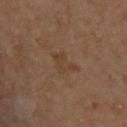  biopsy_status: not biopsied; imaged during a skin examination
  image:
    source: total-body photography crop
    field_of_view_mm: 15
  lighting: cross-polarized
  automated_metrics:
    area_mm2_approx: 5.0
    eccentricity: 0.75
    cielab_L: 40
    cielab_a: 16
    cielab_b: 30
    vs_skin_darker_L: 5.0
    vs_skin_contrast_norm: 5.5
    border_irregularity_0_10: 6.0
    color_variation_0_10: 1.5
  site: upper back
  patient:
    sex: male
    age_approx: 65
  lesion_size:
    long_diameter_mm_approx: 3.5